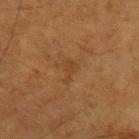The lesion was photographed on a routine skin check and not biopsied; there is no pathology result.